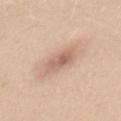site: mid back
lighting: white-light
image:
  source: total-body photography crop
  field_of_view_mm: 15
lesion_size:
  long_diameter_mm_approx: 5.5
patient:
  sex: female
  age_approx: 40
automated_metrics:
  area_mm2_approx: 9.0
  shape_asymmetry: 0.15
  color_variation_0_10: 4.0
  nevus_likeness_0_100: 40
  lesion_detection_confidence_0_100: 100